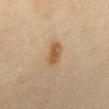This lesion was catalogued during total-body skin photography and was not selected for biopsy. Automated tile analysis of the lesion measured border irregularity of about 2 on a 0–10 scale. It also reported a classifier nevus-likeness of about 95/100. The lesion is on the abdomen. A female patient approximately 80 years of age. Cropped from a whole-body photographic skin survey; the tile spans about 15 mm. The tile uses cross-polarized illumination.